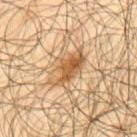No biopsy was performed on this lesion — it was imaged during a full skin examination and was not determined to be concerning.